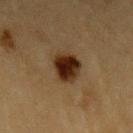Captured during whole-body skin photography for melanoma surveillance; the lesion was not biopsied. A male subject aged 83 or older. On the left upper arm. Approximately 3.5 mm at its widest. Cropped from a whole-body photographic skin survey; the tile spans about 15 mm. The tile uses cross-polarized illumination.